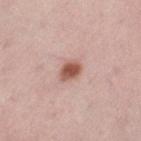On the left thigh. This is a white-light tile. A female subject, in their mid-40s. Cropped from a whole-body photographic skin survey; the tile spans about 15 mm.